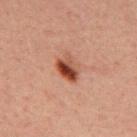Impression:
Captured during whole-body skin photography for melanoma surveillance; the lesion was not biopsied.
Clinical summary:
A male patient, aged approximately 30. The tile uses cross-polarized illumination. From the back. A 15 mm close-up tile from a total-body photography series done for melanoma screening. About 3.5 mm across.